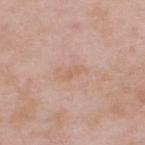site: the upper back
image source: total-body-photography crop, ~15 mm field of view
subject: male, aged 53–57